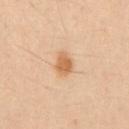• diameter — ~2.5 mm (longest diameter)
• lighting — cross-polarized illumination
• subject — male, aged 38 to 42
• image — 15 mm crop, total-body photography
• location — the front of the torso